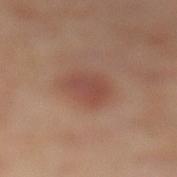Part of a total-body skin-imaging series; this lesion was reviewed on a skin check and was not flagged for biopsy.